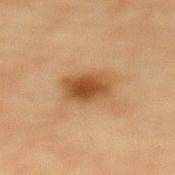Recorded during total-body skin imaging; not selected for excision or biopsy.
A female patient, roughly 80 years of age.
This image is a 15 mm lesion crop taken from a total-body photograph.
The lesion is located on the mid back.